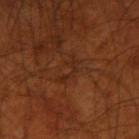follow-up — total-body-photography surveillance lesion; no biopsy | lighting — cross-polarized | location — the right upper arm | subject — male, aged 68 to 72 | lesion diameter — about 3.5 mm | image source — ~15 mm tile from a whole-body skin photo | automated lesion analysis — an average lesion color of about L≈25 a*≈21 b*≈28 (CIELAB), about 4 CIELAB-L* units darker than the surrounding skin, and a lesion-to-skin contrast of about 5 (normalized; higher = more distinct).